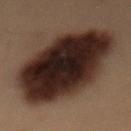Captured during whole-body skin photography for melanoma surveillance; the lesion was not biopsied. This is a cross-polarized tile. The lesion-visualizer software estimated an area of roughly 65 mm² and an outline eccentricity of about 0.9 (0 = round, 1 = elongated). Approximately 13.5 mm at its widest. Located on the mid back. A 15 mm close-up extracted from a 3D total-body photography capture. A male subject, aged 53–57.